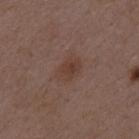Q: Where on the body is the lesion?
A: the mid back
Q: What did automated image analysis measure?
A: a lesion area of about 6.5 mm², a shape eccentricity near 0.75, and a shape-asymmetry score of about 0.2 (0 = symmetric); an average lesion color of about L≈39 a*≈18 b*≈24 (CIELAB), roughly 6 lightness units darker than nearby skin, and a lesion-to-skin contrast of about 6 (normalized; higher = more distinct); a border-irregularity rating of about 2/10, a within-lesion color-variation index near 2.5/10, and a peripheral color-asymmetry measure near 0.5
Q: What kind of image is this?
A: ~15 mm tile from a whole-body skin photo
Q: How large is the lesion?
A: about 3.5 mm
Q: Patient demographics?
A: male, approximately 50 years of age